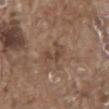biopsy_status: not biopsied; imaged during a skin examination
patient:
  sex: male
  age_approx: 80
site: mid back
image:
  source: total-body photography crop
  field_of_view_mm: 15